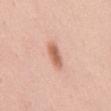notes: catalogued during a skin exam; not biopsied
anatomic site: the mid back
patient: female, aged 48–52
automated metrics: an average lesion color of about L≈63 a*≈24 b*≈31 (CIELAB) and a normalized border contrast of about 8
image: 15 mm crop, total-body photography
illumination: white-light illumination